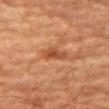<case>
<biopsy_status>not biopsied; imaged during a skin examination</biopsy_status>
<site>right thigh</site>
<lighting>cross-polarized</lighting>
<patient>
  <sex>male</sex>
  <age_approx>80</age_approx>
</patient>
<lesion_size>
  <long_diameter_mm_approx>3.5</long_diameter_mm_approx>
</lesion_size>
<automated_metrics>
  <cielab_L>38</cielab_L>
  <cielab_a>22</cielab_a>
  <cielab_b>31</cielab_b>
  <vs_skin_darker_L>8.0</vs_skin_darker_L>
  <vs_skin_contrast_norm>7.0</vs_skin_contrast_norm>
  <nevus_likeness_0_100>40</nevus_likeness_0_100>
  <lesion_detection_confidence_0_100>100</lesion_detection_confidence_0_100>
</automated_metrics>
<image>
  <source>total-body photography crop</source>
  <field_of_view_mm>15</field_of_view_mm>
</image>
</case>